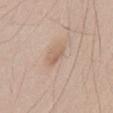No biopsy was performed on this lesion — it was imaged during a full skin examination and was not determined to be concerning. A male subject, aged 58–62. The lesion-visualizer software estimated a lesion area of about 3 mm², a shape eccentricity near 0.8, and a shape-asymmetry score of about 0.25 (0 = symmetric). The software also gave a border-irregularity index near 3/10, a within-lesion color-variation index near 1/10, and radial color variation of about 0.5. A 15 mm close-up extracted from a 3D total-body photography capture. About 2.5 mm across. Captured under white-light illumination. On the lower back.